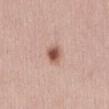Context:
The lesion is on the abdomen. The tile uses white-light illumination. A close-up tile cropped from a whole-body skin photograph, about 15 mm across. A female subject in their mid-40s. Automated tile analysis of the lesion measured a mean CIELAB color near L≈56 a*≈22 b*≈27, a lesion–skin lightness drop of about 14, and a normalized border contrast of about 9.